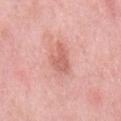Q: Is there a histopathology result?
A: catalogued during a skin exam; not biopsied
Q: How was this image acquired?
A: 15 mm crop, total-body photography
Q: How large is the lesion?
A: about 4 mm
Q: Where on the body is the lesion?
A: the mid back
Q: What lighting was used for the tile?
A: white-light
Q: Patient demographics?
A: male, aged around 55